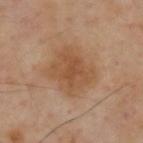Imaged during a routine full-body skin examination; the lesion was not biopsied and no histopathology is available.
The tile uses cross-polarized illumination.
A male subject, about 55 years old.
The lesion's longest dimension is about 5 mm.
This image is a 15 mm lesion crop taken from a total-body photograph.
On the upper back.